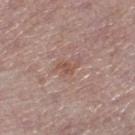Clinical summary: This is a white-light tile. Measured at roughly 2.5 mm in maximum diameter. A female subject, aged 63–67. A region of skin cropped from a whole-body photographic capture, roughly 15 mm wide. The lesion is located on the right lower leg. Automated image analysis of the tile measured a footprint of about 3 mm², an eccentricity of roughly 0.75, and a shape-asymmetry score of about 0.6 (0 = symmetric). It also reported roughly 7 lightness units darker than nearby skin and a normalized lesion–skin contrast near 6.